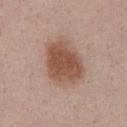Clinical impression:
The lesion was photographed on a routine skin check and not biopsied; there is no pathology result.
Acquisition and patient details:
A male subject, aged 33 to 37. The lesion-visualizer software estimated a footprint of about 19 mm² and an outline eccentricity of about 0.65 (0 = round, 1 = elongated). The software also gave an automated nevus-likeness rating near 100 out of 100 and a detector confidence of about 100 out of 100 that the crop contains a lesion. Located on the chest. A 15 mm close-up extracted from a 3D total-body photography capture. Imaged with white-light lighting. Measured at roughly 5.5 mm in maximum diameter.